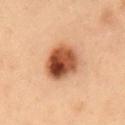<tbp_lesion>
  <biopsy_status>not biopsied; imaged during a skin examination</biopsy_status>
  <lighting>cross-polarized</lighting>
  <lesion_size>
    <long_diameter_mm_approx>4.5</long_diameter_mm_approx>
  </lesion_size>
  <automated_metrics>
    <area_mm2_approx>15.0</area_mm2_approx>
    <shape_asymmetry>0.15</shape_asymmetry>
    <cielab_L>42</cielab_L>
    <cielab_a>22</cielab_a>
    <cielab_b>30</cielab_b>
    <vs_skin_darker_L>17.0</vs_skin_darker_L>
    <vs_skin_contrast_norm>12.5</vs_skin_contrast_norm>
    <border_irregularity_0_10>1.5</border_irregularity_0_10>
  </automated_metrics>
  <site>front of the torso</site>
  <patient>
    <sex>male</sex>
    <age_approx>55</age_approx>
  </patient>
  <image>
    <source>total-body photography crop</source>
    <field_of_view_mm>15</field_of_view_mm>
  </image>
</tbp_lesion>